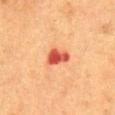Imaged during a routine full-body skin examination; the lesion was not biopsied and no histopathology is available. A female patient aged 48–52. The tile uses cross-polarized illumination. About 3 mm across. From the abdomen. Cropped from a total-body skin-imaging series; the visible field is about 15 mm.